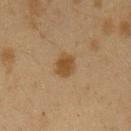{
  "biopsy_status": "not biopsied; imaged during a skin examination",
  "site": "left upper arm",
  "patient": {
    "sex": "female",
    "age_approx": 40
  },
  "lighting": "cross-polarized",
  "image": {
    "source": "total-body photography crop",
    "field_of_view_mm": 15
  },
  "automated_metrics": {
    "cielab_L": 39,
    "cielab_a": 14,
    "cielab_b": 31,
    "vs_skin_darker_L": 8.0,
    "vs_skin_contrast_norm": 8.5,
    "border_irregularity_0_10": 2.0,
    "color_variation_0_10": 1.5
  },
  "lesion_size": {
    "long_diameter_mm_approx": 2.5
  }
}